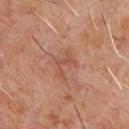Impression: Part of a total-body skin-imaging series; this lesion was reviewed on a skin check and was not flagged for biopsy. Context: A close-up tile cropped from a whole-body skin photograph, about 15 mm across. On the front of the torso. A male subject in their 60s. Captured under cross-polarized illumination.